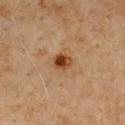The lesion was tiled from a total-body skin photograph and was not biopsied. A male patient, in their 60s. On the chest. A 15 mm crop from a total-body photograph taken for skin-cancer surveillance.